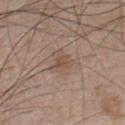notes: catalogued during a skin exam; not biopsied
imaging modality: ~15 mm crop, total-body skin-cancer survey
illumination: white-light
image-analysis metrics: a border-irregularity rating of about 3.5/10 and peripheral color asymmetry of about 1; a nevus-likeness score of about 0/100 and a detector confidence of about 100 out of 100 that the crop contains a lesion
anatomic site: the chest
lesion diameter: about 3.5 mm
patient: male, aged 48–52